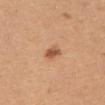follow-up — imaged on a skin check; not biopsied | patient — female, aged 43–47 | image — total-body-photography crop, ~15 mm field of view | anatomic site — the upper back | size — ~2.5 mm (longest diameter).